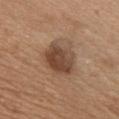| feature | finding |
|---|---|
| biopsy status | total-body-photography surveillance lesion; no biopsy |
| tile lighting | white-light illumination |
| subject | male, about 50 years old |
| diameter | ~4.5 mm (longest diameter) |
| imaging modality | total-body-photography crop, ~15 mm field of view |
| location | the front of the torso |